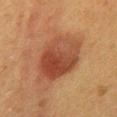Imaged during a routine full-body skin examination; the lesion was not biopsied and no histopathology is available. The subject is a female approximately 50 years of age. A roughly 15 mm field-of-view crop from a total-body skin photograph. The recorded lesion diameter is about 7.5 mm. From the mid back.